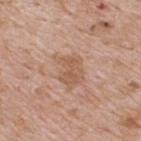| field | value |
|---|---|
| notes | total-body-photography surveillance lesion; no biopsy |
| lighting | white-light |
| site | the back |
| image | 15 mm crop, total-body photography |
| diameter | ≈4 mm |
| patient | male, in their mid- to late 60s |